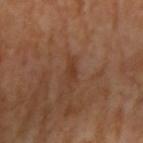The lesion is located on the right upper arm. A 15 mm close-up tile from a total-body photography series done for melanoma screening.